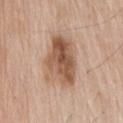Notes:
- notes — no biopsy performed (imaged during a skin exam)
- anatomic site — the head or neck
- imaging modality — 15 mm crop, total-body photography
- subject — male, aged approximately 80
- lighting — white-light
- size — ~6.5 mm (longest diameter)